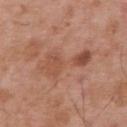Cropped from a whole-body photographic skin survey; the tile spans about 15 mm. A male subject aged 53 to 57. Captured under white-light illumination. The lesion is on the upper back.